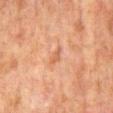Q: Was this lesion biopsied?
A: imaged on a skin check; not biopsied
Q: How was this image acquired?
A: 15 mm crop, total-body photography
Q: Where on the body is the lesion?
A: the mid back
Q: What are the patient's age and sex?
A: male, aged approximately 60
Q: How was the tile lit?
A: cross-polarized
Q: What is the lesion's diameter?
A: ≈2.5 mm